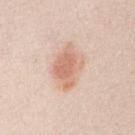No biopsy was performed on this lesion — it was imaged during a full skin examination and was not determined to be concerning.